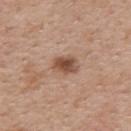Notes:
– biopsy status: no biopsy performed (imaged during a skin exam)
– acquisition: 15 mm crop, total-body photography
– body site: the upper back
– patient: female, approximately 40 years of age
– diameter: ~3 mm (longest diameter)